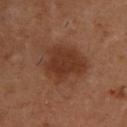patient=male, in their mid-50s
lesion diameter=≈5.5 mm
illumination=cross-polarized
site=the upper back
image source=~15 mm crop, total-body skin-cancer survey